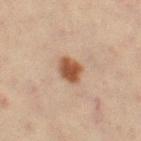Case summary:
– notes — total-body-photography surveillance lesion; no biopsy
– patient — female, in their mid- to late 40s
– automated metrics — a mean CIELAB color near L≈45 a*≈19 b*≈29 and a normalized lesion–skin contrast near 10.5; a detector confidence of about 100 out of 100 that the crop contains a lesion
– diameter — about 3 mm
– anatomic site — the left thigh
– image source — ~15 mm crop, total-body skin-cancer survey